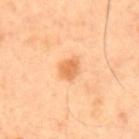biopsy status — no biopsy performed (imaged during a skin exam) | lighting — cross-polarized | anatomic site — the back | imaging modality — total-body-photography crop, ~15 mm field of view | TBP lesion metrics — an average lesion color of about L≈69 a*≈27 b*≈45 (CIELAB), roughly 11 lightness units darker than nearby skin, and a lesion-to-skin contrast of about 7 (normalized; higher = more distinct); border irregularity of about 2 on a 0–10 scale, internal color variation of about 2 on a 0–10 scale, and radial color variation of about 1; a lesion-detection confidence of about 100/100 | lesion diameter — ≈3 mm | subject — male, in their mid- to late 60s.